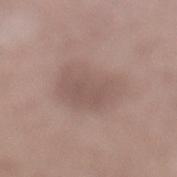follow-up: catalogued during a skin exam; not biopsied
anatomic site: the left lower leg
patient: male, aged approximately 50
acquisition: total-body-photography crop, ~15 mm field of view
automated metrics: an area of roughly 21 mm², an eccentricity of roughly 0.65, and two-axis asymmetry of about 0.2; an automated nevus-likeness rating near 0 out of 100 and lesion-presence confidence of about 100/100